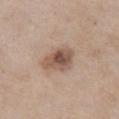<lesion>
<biopsy_status>not biopsied; imaged during a skin examination</biopsy_status>
<automated_metrics>
  <area_mm2_approx>9.5</area_mm2_approx>
  <shape_asymmetry>0.3</shape_asymmetry>
</automated_metrics>
<lighting>white-light</lighting>
<image>
  <source>total-body photography crop</source>
  <field_of_view_mm>15</field_of_view_mm>
</image>
<lesion_size>
  <long_diameter_mm_approx>4.0</long_diameter_mm_approx>
</lesion_size>
<site>front of the torso</site>
<patient>
  <sex>female</sex>
  <age_approx>40</age_approx>
</patient>
</lesion>